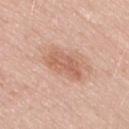* notes · no biopsy performed (imaged during a skin exam)
* site · the right thigh
* image · 15 mm crop, total-body photography
* lighting · white-light illumination
* patient · female, in their 60s
* lesion size · about 4.5 mm